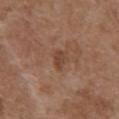Part of a total-body skin-imaging series; this lesion was reviewed on a skin check and was not flagged for biopsy.
A male patient, in their mid-70s.
Automated image analysis of the tile measured a lesion area of about 3.5 mm², an outline eccentricity of about 0.8 (0 = round, 1 = elongated), and a symmetry-axis asymmetry near 0.35. The analysis additionally found a border-irregularity index near 3.5/10, internal color variation of about 1 on a 0–10 scale, and radial color variation of about 0.5.
Longest diameter approximately 2.5 mm.
A lesion tile, about 15 mm wide, cut from a 3D total-body photograph.
This is a white-light tile.
Located on the abdomen.